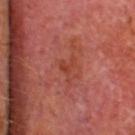Clinical impression:
The lesion was photographed on a routine skin check and not biopsied; there is no pathology result.
Background:
A close-up tile cropped from a whole-body skin photograph, about 15 mm across. Longest diameter approximately 3 mm. Automated image analysis of the tile measured a shape-asymmetry score of about 0.6 (0 = symmetric). And it measured about 6 CIELAB-L* units darker than the surrounding skin and a normalized border contrast of about 6. The software also gave a border-irregularity rating of about 6.5/10, internal color variation of about 0 on a 0–10 scale, and radial color variation of about 0. The software also gave a classifier nevus-likeness of about 0/100 and a detector confidence of about 100 out of 100 that the crop contains a lesion. Captured under cross-polarized illumination. A male subject roughly 65 years of age. The lesion is located on the head or neck.